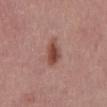Q: Is there a histopathology result?
A: no biopsy performed (imaged during a skin exam)
Q: Illumination type?
A: white-light
Q: Automated lesion metrics?
A: border irregularity of about 3 on a 0–10 scale, a within-lesion color-variation index near 3.5/10, and a peripheral color-asymmetry measure near 1; an automated nevus-likeness rating near 95 out of 100
Q: Who is the patient?
A: male, aged 53 to 57
Q: What is the imaging modality?
A: total-body-photography crop, ~15 mm field of view
Q: What is the lesion's diameter?
A: about 4 mm
Q: What is the anatomic site?
A: the abdomen